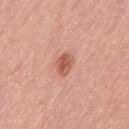Part of a total-body skin-imaging series; this lesion was reviewed on a skin check and was not flagged for biopsy. A region of skin cropped from a whole-body photographic capture, roughly 15 mm wide. Longest diameter approximately 3 mm. An algorithmic analysis of the crop reported a lesion color around L≈57 a*≈27 b*≈30 in CIELAB, roughly 12 lightness units darker than nearby skin, and a lesion-to-skin contrast of about 7.5 (normalized; higher = more distinct). And it measured lesion-presence confidence of about 100/100. A female subject, aged around 50. The lesion is on the left thigh. The tile uses white-light illumination.